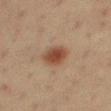No biopsy was performed on this lesion — it was imaged during a full skin examination and was not determined to be concerning. A female patient, approximately 20 years of age. On the leg. A region of skin cropped from a whole-body photographic capture, roughly 15 mm wide.